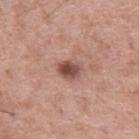<case>
  <biopsy_status>not biopsied; imaged during a skin examination</biopsy_status>
  <lighting>white-light</lighting>
  <patient>
    <sex>male</sex>
    <age_approx>55</age_approx>
  </patient>
  <lesion_size>
    <long_diameter_mm_approx>3.0</long_diameter_mm_approx>
  </lesion_size>
  <image>
    <source>total-body photography crop</source>
    <field_of_view_mm>15</field_of_view_mm>
  </image>
  <automated_metrics>
    <cielab_L>49</cielab_L>
    <cielab_a>22</cielab_a>
    <cielab_b>26</cielab_b>
    <vs_skin_darker_L>14.0</vs_skin_darker_L>
  </automated_metrics>
</case>